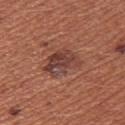Assessment: Imaged during a routine full-body skin examination; the lesion was not biopsied and no histopathology is available. Acquisition and patient details: The lesion is located on the right upper arm. A 15 mm close-up extracted from a 3D total-body photography capture. A female subject about 35 years old.